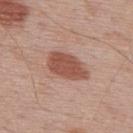Recorded during total-body skin imaging; not selected for excision or biopsy. A male subject aged 68 to 72. The lesion is on the upper back. A 15 mm close-up tile from a total-body photography series done for melanoma screening.